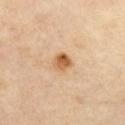Recorded during total-body skin imaging; not selected for excision or biopsy. The patient is a female aged approximately 65. Cropped from a whole-body photographic skin survey; the tile spans about 15 mm. On the chest.